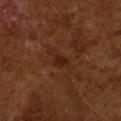Assessment: This lesion was catalogued during total-body skin photography and was not selected for biopsy. Acquisition and patient details: The tile uses cross-polarized illumination. A male patient aged 63–67. A region of skin cropped from a whole-body photographic capture, roughly 15 mm wide. Measured at roughly 3 mm in maximum diameter. An algorithmic analysis of the crop reported an area of roughly 3.5 mm² and a shape eccentricity near 0.85. The analysis additionally found a lesion color around L≈23 a*≈20 b*≈26 in CIELAB. The analysis additionally found an automated nevus-likeness rating near 5 out of 100 and a detector confidence of about 100 out of 100 that the crop contains a lesion.